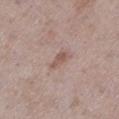This lesion was catalogued during total-body skin photography and was not selected for biopsy.
A roughly 15 mm field-of-view crop from a total-body skin photograph.
The tile uses white-light illumination.
About 3 mm across.
A female patient, aged approximately 60.
The lesion is located on the left lower leg.